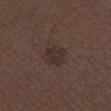No biopsy was performed on this lesion — it was imaged during a full skin examination and was not determined to be concerning. The lesion is on the right lower leg. This image is a 15 mm lesion crop taken from a total-body photograph. This is a white-light tile. A male patient, aged around 50. Measured at roughly 3 mm in maximum diameter.